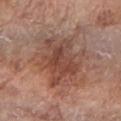  biopsy_status: not biopsied; imaged during a skin examination
  patient:
    sex: female
    age_approx: 75
  image:
    source: total-body photography crop
    field_of_view_mm: 15
  site: arm
  lighting: white-light